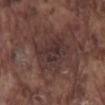{"biopsy_status": "not biopsied; imaged during a skin examination", "patient": {"sex": "male", "age_approx": 75}, "image": {"source": "total-body photography crop", "field_of_view_mm": 15}, "lesion_size": {"long_diameter_mm_approx": 5.5}, "lighting": "white-light", "site": "mid back"}